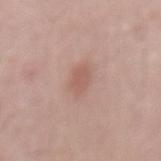notes: imaged on a skin check; not biopsied
patient: male, aged 48–52
image: ~15 mm tile from a whole-body skin photo
anatomic site: the lower back